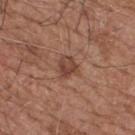Assessment: Captured during whole-body skin photography for melanoma surveillance; the lesion was not biopsied. Clinical summary: Imaged with white-light lighting. A lesion tile, about 15 mm wide, cut from a 3D total-body photograph. The patient is a male in their mid- to late 70s. Located on the upper back. The lesion's longest dimension is about 2.5 mm.